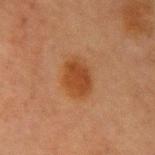Imaged during a routine full-body skin examination; the lesion was not biopsied and no histopathology is available.
The lesion is located on the right upper arm.
Cropped from a total-body skin-imaging series; the visible field is about 15 mm.
Longest diameter approximately 4 mm.
Captured under cross-polarized illumination.
A male patient in their mid- to late 60s.